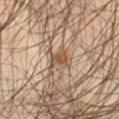No biopsy was performed on this lesion — it was imaged during a full skin examination and was not determined to be concerning.
A male patient, approximately 40 years of age.
The total-body-photography lesion software estimated a lesion area of about 3.5 mm² and an outline eccentricity of about 0.7 (0 = round, 1 = elongated). The analysis additionally found a lesion color around L≈51 a*≈18 b*≈32 in CIELAB, roughly 10 lightness units darker than nearby skin, and a lesion-to-skin contrast of about 8 (normalized; higher = more distinct). It also reported a border-irregularity rating of about 2.5/10. The analysis additionally found a nevus-likeness score of about 85/100 and lesion-presence confidence of about 100/100.
The lesion's longest dimension is about 2.5 mm.
A region of skin cropped from a whole-body photographic capture, roughly 15 mm wide.
On the front of the torso.